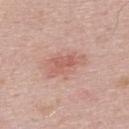<case>
  <biopsy_status>not biopsied; imaged during a skin examination</biopsy_status>
  <image>
    <source>total-body photography crop</source>
    <field_of_view_mm>15</field_of_view_mm>
  </image>
  <patient>
    <sex>male</sex>
    <age_approx>25</age_approx>
  </patient>
  <site>upper back</site>
</case>